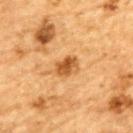subject: male, aged 83 or over; acquisition: 15 mm crop, total-body photography; location: the upper back; tile lighting: cross-polarized; lesion diameter: ~3 mm (longest diameter).